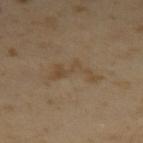No biopsy was performed on this lesion — it was imaged during a full skin examination and was not determined to be concerning. This is a cross-polarized tile. Cropped from a whole-body photographic skin survey; the tile spans about 15 mm. About 5 mm across. A male subject about 65 years old.